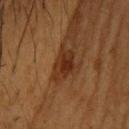biopsy_status: not biopsied; imaged during a skin examination
automated_metrics:
  area_mm2_approx: 6.5
  eccentricity: 0.8
  shape_asymmetry: 0.3
  cielab_L: 27
  cielab_a: 20
  cielab_b: 29
  vs_skin_darker_L: 9.0
  vs_skin_contrast_norm: 9.5
  nevus_likeness_0_100: 65
  lesion_detection_confidence_0_100: 100
image:
  source: total-body photography crop
  field_of_view_mm: 15
lighting: cross-polarized
site: head or neck
lesion_size:
  long_diameter_mm_approx: 4.0
patient:
  sex: male
  age_approx: 40